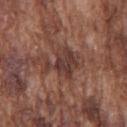The lesion was tiled from a total-body skin photograph and was not biopsied.
The lesion-visualizer software estimated an area of roughly 9 mm² and two-axis asymmetry of about 0.5.
The patient is a male in their mid- to late 70s.
A 15 mm close-up tile from a total-body photography series done for melanoma screening.
Located on the chest.